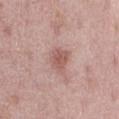Assessment:
The lesion was photographed on a routine skin check and not biopsied; there is no pathology result.
Acquisition and patient details:
A 15 mm crop from a total-body photograph taken for skin-cancer surveillance. Measured at roughly 3 mm in maximum diameter. A male patient, aged 78–82. The total-body-photography lesion software estimated a footprint of about 6 mm², an eccentricity of roughly 0.7, and two-axis asymmetry of about 0.25. And it measured a lesion color around L≈56 a*≈22 b*≈24 in CIELAB. The lesion is on the abdomen. The tile uses white-light illumination.